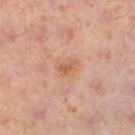The lesion is located on the left lower leg.
Automated image analysis of the tile measured a lesion color around L≈59 a*≈24 b*≈33 in CIELAB, roughly 8 lightness units darker than nearby skin, and a normalized border contrast of about 6. It also reported a classifier nevus-likeness of about 5/100 and a lesion-detection confidence of about 100/100.
A female subject, aged 38–42.
Longest diameter approximately 3 mm.
A roughly 15 mm field-of-view crop from a total-body skin photograph.